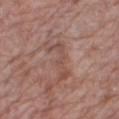Recorded during total-body skin imaging; not selected for excision or biopsy.
Imaged with white-light lighting.
Cropped from a whole-body photographic skin survey; the tile spans about 15 mm.
Measured at roughly 5 mm in maximum diameter.
An algorithmic analysis of the crop reported an average lesion color of about L≈49 a*≈20 b*≈25 (CIELAB), a lesion–skin lightness drop of about 7, and a normalized border contrast of about 5. And it measured a border-irregularity index near 10/10 and a within-lesion color-variation index near 2/10. The software also gave a classifier nevus-likeness of about 0/100 and a lesion-detection confidence of about 85/100.
A female patient aged approximately 70.
From the right thigh.